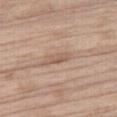follow-up: catalogued during a skin exam; not biopsied | body site: the right thigh | patient: male, roughly 70 years of age | image: 15 mm crop, total-body photography | illumination: white-light illumination | automated metrics: a footprint of about 4 mm² and a symmetry-axis asymmetry near 0.4; a classifier nevus-likeness of about 0/100 and a lesion-detection confidence of about 70/100 | diameter: ≈3.5 mm.